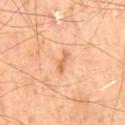| field | value |
|---|---|
| biopsy status | imaged on a skin check; not biopsied |
| image source | 15 mm crop, total-body photography |
| subject | male, roughly 70 years of age |
| illumination | cross-polarized illumination |
| anatomic site | the right thigh |